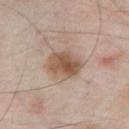Assessment: Recorded during total-body skin imaging; not selected for excision or biopsy. Background: A male patient aged 53–57. Located on the chest. A 15 mm crop from a total-body photograph taken for skin-cancer surveillance.